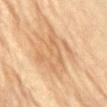lighting = cross-polarized; acquisition = ~15 mm tile from a whole-body skin photo; patient = female, aged 78 to 82; anatomic site = the abdomen.